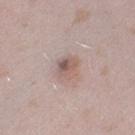biopsy status=catalogued during a skin exam; not biopsied | acquisition=total-body-photography crop, ~15 mm field of view | site=the left thigh | tile lighting=white-light illumination | size=about 2.5 mm | patient=female, approximately 25 years of age | image-analysis metrics=an average lesion color of about L≈57 a*≈17 b*≈22 (CIELAB); border irregularity of about 2 on a 0–10 scale and a color-variation rating of about 7/10; a classifier nevus-likeness of about 50/100 and a detector confidence of about 95 out of 100 that the crop contains a lesion.